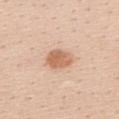Captured during whole-body skin photography for melanoma surveillance; the lesion was not biopsied.
The patient is a female aged 23–27.
Approximately 3.5 mm at its widest.
Captured under white-light illumination.
Cropped from a whole-body photographic skin survey; the tile spans about 15 mm.
On the chest.